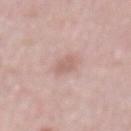A male subject, aged 48–52. The lesion-visualizer software estimated a lesion color around L≈62 a*≈20 b*≈24 in CIELAB, a lesion–skin lightness drop of about 8, and a normalized lesion–skin contrast near 5.5. And it measured a border-irregularity index near 3/10 and radial color variation of about 0.5. It also reported a classifier nevus-likeness of about 0/100. A close-up tile cropped from a whole-body skin photograph, about 15 mm across. The lesion is located on the abdomen. The lesion's longest dimension is about 3 mm. The tile uses white-light illumination.